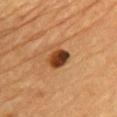Q: Was this lesion biopsied?
A: no biopsy performed (imaged during a skin exam)
Q: What is the imaging modality?
A: 15 mm crop, total-body photography
Q: Who is the patient?
A: male, aged 83 or older
Q: What is the anatomic site?
A: the chest
Q: How was the tile lit?
A: cross-polarized illumination